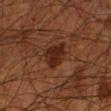{"biopsy_status": "not biopsied; imaged during a skin examination", "patient": {"sex": "male", "age_approx": 65}, "site": "arm", "image": {"source": "total-body photography crop", "field_of_view_mm": 15}}